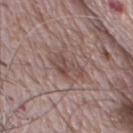| field | value |
|---|---|
| follow-up | imaged on a skin check; not biopsied |
| image-analysis metrics | a lesion–skin lightness drop of about 9 and a lesion-to-skin contrast of about 6.5 (normalized; higher = more distinct); a nevus-likeness score of about 0/100 and a lesion-detection confidence of about 55/100 |
| anatomic site | the chest |
| acquisition | 15 mm crop, total-body photography |
| patient | male, aged 68–72 |
| lighting | white-light |